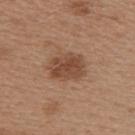Q: Was this lesion biopsied?
A: no biopsy performed (imaged during a skin exam)
Q: What did automated image analysis measure?
A: a shape eccentricity near 0.7; a classifier nevus-likeness of about 45/100 and lesion-presence confidence of about 100/100
Q: Where on the body is the lesion?
A: the upper back
Q: Lesion size?
A: ~4.5 mm (longest diameter)
Q: What are the patient's age and sex?
A: female, aged around 40
Q: What kind of image is this?
A: total-body-photography crop, ~15 mm field of view